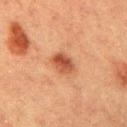Clinical impression:
Recorded during total-body skin imaging; not selected for excision or biopsy.
Background:
Imaged with cross-polarized lighting. A roughly 15 mm field-of-view crop from a total-body skin photograph. A female patient, in their mid-50s. The lesion-visualizer software estimated a lesion area of about 5.5 mm² and two-axis asymmetry of about 0.15. The software also gave lesion-presence confidence of about 100/100. The lesion is on the right upper arm. The lesion's longest dimension is about 3 mm.